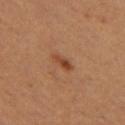Q: Is there a histopathology result?
A: catalogued during a skin exam; not biopsied
Q: What is the anatomic site?
A: the left thigh
Q: What did automated image analysis measure?
A: an outline eccentricity of about 0.9 (0 = round, 1 = elongated) and a symmetry-axis asymmetry near 0.25; an average lesion color of about L≈45 a*≈25 b*≈34 (CIELAB), roughly 10 lightness units darker than nearby skin, and a normalized border contrast of about 7.5; a classifier nevus-likeness of about 80/100 and a lesion-detection confidence of about 100/100
Q: Patient demographics?
A: female, aged around 55
Q: Illumination type?
A: cross-polarized
Q: How was this image acquired?
A: ~15 mm tile from a whole-body skin photo
Q: Lesion size?
A: ≈2.5 mm